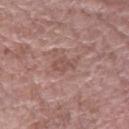workup: imaged on a skin check; not biopsied
image-analysis metrics: an eccentricity of roughly 0.85; a mean CIELAB color near L≈51 a*≈21 b*≈23 and a normalized border contrast of about 5.5; peripheral color asymmetry of about 0.5; a classifier nevus-likeness of about 0/100
lesion diameter: ~3 mm (longest diameter)
acquisition: total-body-photography crop, ~15 mm field of view
anatomic site: the right forearm
patient: male, in their mid-50s